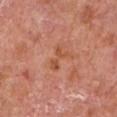Captured during whole-body skin photography for melanoma surveillance; the lesion was not biopsied. Located on the chest. Imaged with white-light lighting. A male subject approximately 65 years of age. The lesion's longest dimension is about 3 mm. A 15 mm crop from a total-body photograph taken for skin-cancer surveillance. An algorithmic analysis of the crop reported a footprint of about 4.5 mm², an eccentricity of roughly 0.85, and a symmetry-axis asymmetry near 0.35. And it measured a lesion–skin lightness drop of about 7.